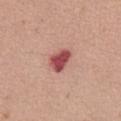Q: Was this lesion biopsied?
A: catalogued during a skin exam; not biopsied
Q: Patient demographics?
A: female, about 65 years old
Q: What lighting was used for the tile?
A: white-light illumination
Q: What kind of image is this?
A: total-body-photography crop, ~15 mm field of view
Q: Where on the body is the lesion?
A: the abdomen
Q: What did automated image analysis measure?
A: a lesion area of about 6 mm², an outline eccentricity of about 0.7 (0 = round, 1 = elongated), and two-axis asymmetry of about 0.2; a color-variation rating of about 2.5/10 and radial color variation of about 1; an automated nevus-likeness rating near 0 out of 100
Q: What is the lesion's diameter?
A: about 3 mm